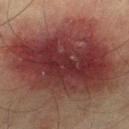This lesion was catalogued during total-body skin photography and was not selected for biopsy.
Automated image analysis of the tile measured a color-variation rating of about 7.5/10 and peripheral color asymmetry of about 2.5. The software also gave a nevus-likeness score of about 30/100 and a detector confidence of about 100 out of 100 that the crop contains a lesion.
This is a cross-polarized tile.
The recorded lesion diameter is about 12.5 mm.
The subject is a male in their mid-70s.
Cropped from a whole-body photographic skin survey; the tile spans about 15 mm.
From the left thigh.